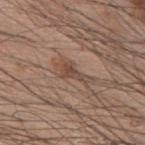  biopsy_status: not biopsied; imaged during a skin examination
  automated_metrics:
    border_irregularity_0_10: 6.0
    color_variation_0_10: 2.0
    peripheral_color_asymmetry: 0.5
  site: upper back
  lesion_size:
    long_diameter_mm_approx: 3.5
  lighting: white-light
  patient:
    sex: male
    age_approx: 60
  image:
    source: total-body photography crop
    field_of_view_mm: 15